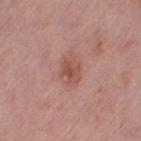Q: Was a biopsy performed?
A: no biopsy performed (imaged during a skin exam)
Q: Patient demographics?
A: female, aged 63 to 67
Q: What is the lesion's diameter?
A: ~2.5 mm (longest diameter)
Q: Where on the body is the lesion?
A: the right thigh
Q: What did automated image analysis measure?
A: an average lesion color of about L≈51 a*≈25 b*≈26 (CIELAB) and a lesion-to-skin contrast of about 6.5 (normalized; higher = more distinct)
Q: How was this image acquired?
A: 15 mm crop, total-body photography
Q: Illumination type?
A: white-light